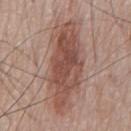This lesion was catalogued during total-body skin photography and was not selected for biopsy. The lesion is on the chest. A male patient, aged approximately 80. A lesion tile, about 15 mm wide, cut from a 3D total-body photograph. Automated tile analysis of the lesion measured a footprint of about 32 mm², a shape eccentricity near 0.95, and two-axis asymmetry of about 0.2. It also reported a border-irregularity rating of about 4/10 and radial color variation of about 1.5. The recorded lesion diameter is about 10.5 mm.